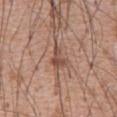notes: total-body-photography surveillance lesion; no biopsy
acquisition: ~15 mm tile from a whole-body skin photo
image-analysis metrics: an area of roughly 4 mm² and an eccentricity of roughly 0.7; a border-irregularity rating of about 5/10, internal color variation of about 2.5 on a 0–10 scale, and a peripheral color-asymmetry measure near 1
subject: male, roughly 60 years of age
size: ~2.5 mm (longest diameter)
location: the abdomen